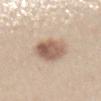Q: Is there a histopathology result?
A: no biopsy performed (imaged during a skin exam)
Q: Patient demographics?
A: female, aged approximately 45
Q: Illumination type?
A: white-light illumination
Q: What is the imaging modality?
A: total-body-photography crop, ~15 mm field of view
Q: What is the anatomic site?
A: the abdomen
Q: How large is the lesion?
A: about 5.5 mm